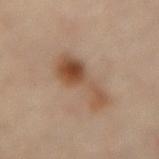Clinical impression:
The lesion was photographed on a routine skin check and not biopsied; there is no pathology result.
Context:
The recorded lesion diameter is about 6 mm. Automated image analysis of the tile measured a normalized border contrast of about 8.5. The analysis additionally found a border-irregularity index near 6.5/10 and a color-variation rating of about 7/10. The analysis additionally found an automated nevus-likeness rating near 80 out of 100 and lesion-presence confidence of about 100/100. The tile uses cross-polarized illumination. Located on the lower back. A female subject, in their 60s. A lesion tile, about 15 mm wide, cut from a 3D total-body photograph.